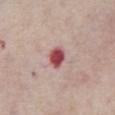Clinical summary:
On the chest. Longest diameter approximately 3 mm. A male subject approximately 85 years of age or older. The total-body-photography lesion software estimated a footprint of about 5 mm², a shape eccentricity near 0.6, and a shape-asymmetry score of about 0.15 (0 = symmetric). The software also gave a lesion color around L≈50 a*≈32 b*≈21 in CIELAB and roughly 18 lightness units darker than nearby skin. A lesion tile, about 15 mm wide, cut from a 3D total-body photograph.